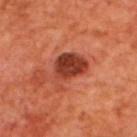Imaged during a routine full-body skin examination; the lesion was not biopsied and no histopathology is available. This is a cross-polarized tile. A male patient, roughly 70 years of age. The lesion is on the upper back. The lesion's longest dimension is about 4.5 mm. This image is a 15 mm lesion crop taken from a total-body photograph.